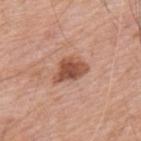<record>
<biopsy_status>not biopsied; imaged during a skin examination</biopsy_status>
<lesion_size>
  <long_diameter_mm_approx>4.0</long_diameter_mm_approx>
</lesion_size>
<image>
  <source>total-body photography crop</source>
  <field_of_view_mm>15</field_of_view_mm>
</image>
<lighting>white-light</lighting>
<patient>
  <sex>male</sex>
  <age_approx>60</age_approx>
</patient>
<site>back</site>
<automated_metrics>
  <area_mm2_approx>8.0</area_mm2_approx>
  <eccentricity>0.75</eccentricity>
  <shape_asymmetry>0.3</shape_asymmetry>
  <cielab_L>52</cielab_L>
  <cielab_a>24</cielab_a>
  <cielab_b>31</cielab_b>
  <vs_skin_darker_L>14.0</vs_skin_darker_L>
</automated_metrics>
</record>